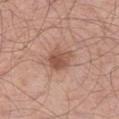Clinical impression: Part of a total-body skin-imaging series; this lesion was reviewed on a skin check and was not flagged for biopsy. Context: This image is a 15 mm lesion crop taken from a total-body photograph. A male patient aged 68–72. Automated tile analysis of the lesion measured a lesion color around L≈53 a*≈22 b*≈28 in CIELAB, about 11 CIELAB-L* units darker than the surrounding skin, and a normalized border contrast of about 7.5. The software also gave a border-irregularity rating of about 2.5/10, a color-variation rating of about 4/10, and a peripheral color-asymmetry measure near 1.5. From the left thigh. Captured under white-light illumination.